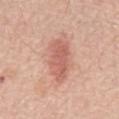Q: Is there a histopathology result?
A: imaged on a skin check; not biopsied
Q: What is the imaging modality?
A: 15 mm crop, total-body photography
Q: What did automated image analysis measure?
A: a border-irregularity rating of about 3/10, a within-lesion color-variation index near 2.5/10, and peripheral color asymmetry of about 1; a classifier nevus-likeness of about 85/100 and a detector confidence of about 100 out of 100 that the crop contains a lesion
Q: Patient demographics?
A: male, aged 68 to 72
Q: How was the tile lit?
A: white-light
Q: What is the anatomic site?
A: the mid back
Q: What is the lesion's diameter?
A: ≈5.5 mm